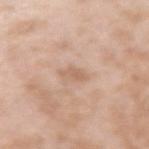Impression: Imaged during a routine full-body skin examination; the lesion was not biopsied and no histopathology is available. Acquisition and patient details: On the right upper arm. A male subject, aged approximately 45. This image is a 15 mm lesion crop taken from a total-body photograph.